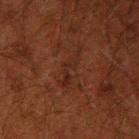Imaged during a routine full-body skin examination; the lesion was not biopsied and no histopathology is available. A close-up tile cropped from a whole-body skin photograph, about 15 mm across. A male patient, in their 50s. The lesion is located on the right upper arm. This is a cross-polarized tile.